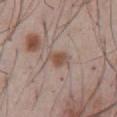Notes:
- notes — no biopsy performed (imaged during a skin exam)
- body site — the front of the torso
- image source — ~15 mm tile from a whole-body skin photo
- size — ~3 mm (longest diameter)
- patient — male, aged 53–57
- lighting — white-light